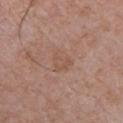The lesion was photographed on a routine skin check and not biopsied; there is no pathology result.
From the chest.
A roughly 15 mm field-of-view crop from a total-body skin photograph.
The subject is a male aged 68–72.